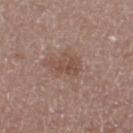Clinical impression:
No biopsy was performed on this lesion — it was imaged during a full skin examination and was not determined to be concerning.
Background:
A male subject aged 73 to 77. Measured at roughly 3.5 mm in maximum diameter. Imaged with white-light lighting. A lesion tile, about 15 mm wide, cut from a 3D total-body photograph. The lesion is located on the left lower leg.